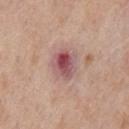<tbp_lesion>
  <biopsy_status>not biopsied; imaged during a skin examination</biopsy_status>
  <image>
    <source>total-body photography crop</source>
    <field_of_view_mm>15</field_of_view_mm>
  </image>
  <patient>
    <sex>male</sex>
    <age_approx>75</age_approx>
  </patient>
  <site>chest</site>
</tbp_lesion>